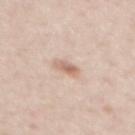<record>
<biopsy_status>not biopsied; imaged during a skin examination</biopsy_status>
<site>back</site>
<image>
  <source>total-body photography crop</source>
  <field_of_view_mm>15</field_of_view_mm>
</image>
<automated_metrics>
  <area_mm2_approx>3.0</area_mm2_approx>
  <eccentricity>0.85</eccentricity>
  <shape_asymmetry>0.3</shape_asymmetry>
  <cielab_L>65</cielab_L>
  <cielab_a>19</cielab_a>
  <cielab_b>28</cielab_b>
  <vs_skin_darker_L>11.0</vs_skin_darker_L>
  <vs_skin_contrast_norm>7.0</vs_skin_contrast_norm>
  <border_irregularity_0_10>3.0</border_irregularity_0_10>
  <color_variation_0_10>4.0</color_variation_0_10>
  <peripheral_color_asymmetry>1.5</peripheral_color_asymmetry>
  <nevus_likeness_0_100>80</nevus_likeness_0_100>
  <lesion_detection_confidence_0_100>100</lesion_detection_confidence_0_100>
</automated_metrics>
<patient>
  <sex>female</sex>
  <age_approx>45</age_approx>
</patient>
<lighting>white-light</lighting>
</record>